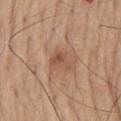{
  "biopsy_status": "not biopsied; imaged during a skin examination",
  "site": "mid back",
  "lighting": "white-light",
  "image": {
    "source": "total-body photography crop",
    "field_of_view_mm": 15
  },
  "automated_metrics": {
    "cielab_L": 52,
    "cielab_a": 20,
    "cielab_b": 30,
    "vs_skin_darker_L": 9.0,
    "nevus_likeness_0_100": 25,
    "lesion_detection_confidence_0_100": 100
  },
  "patient": {
    "sex": "male",
    "age_approx": 65
  },
  "lesion_size": {
    "long_diameter_mm_approx": 3.0
  }
}